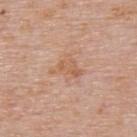Clinical impression: The lesion was photographed on a routine skin check and not biopsied; there is no pathology result. Context: Automated tile analysis of the lesion measured a footprint of about 6.5 mm². The software also gave a lesion–skin lightness drop of about 7 and a normalized border contrast of about 5.5. The analysis additionally found a border-irregularity index near 5/10, a color-variation rating of about 2/10, and a peripheral color-asymmetry measure near 0.5. And it measured an automated nevus-likeness rating near 0 out of 100 and lesion-presence confidence of about 100/100. The subject is a male aged approximately 75. Cropped from a whole-body photographic skin survey; the tile spans about 15 mm. This is a white-light tile. The lesion's longest dimension is about 3 mm. Located on the upper back.